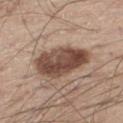No biopsy was performed on this lesion — it was imaged during a full skin examination and was not determined to be concerning. This is a white-light tile. The total-body-photography lesion software estimated an area of roughly 20 mm² and a shape-asymmetry score of about 0.2 (0 = symmetric). The analysis additionally found an average lesion color of about L≈47 a*≈18 b*≈25 (CIELAB), about 16 CIELAB-L* units darker than the surrounding skin, and a normalized border contrast of about 11. And it measured a within-lesion color-variation index near 6/10 and a peripheral color-asymmetry measure near 2.5. The recorded lesion diameter is about 6.5 mm. A roughly 15 mm field-of-view crop from a total-body skin photograph. The lesion is on the left thigh. A male subject roughly 60 years of age.